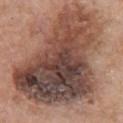workup: no biopsy performed (imaged during a skin exam)
lesion size: ≈15 mm
location: the chest
automated lesion analysis: two-axis asymmetry of about 0.4; a mean CIELAB color near L≈46 a*≈19 b*≈24, a lesion–skin lightness drop of about 16, and a lesion-to-skin contrast of about 11.5 (normalized; higher = more distinct); a border-irregularity rating of about 5.5/10, a within-lesion color-variation index near 10/10, and radial color variation of about 3.5; a detector confidence of about 100 out of 100 that the crop contains a lesion
acquisition: 15 mm crop, total-body photography
subject: female, about 60 years old
tile lighting: white-light illumination